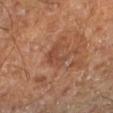{"biopsy_status": "not biopsied; imaged during a skin examination", "automated_metrics": {"area_mm2_approx": 6.0, "shape_asymmetry": 0.5, "border_irregularity_0_10": 6.0, "peripheral_color_asymmetry": 0.5}, "patient": {"age_approx": 65}, "image": {"source": "total-body photography crop", "field_of_view_mm": 15}, "site": "left lower leg", "lighting": "cross-polarized", "lesion_size": {"long_diameter_mm_approx": 3.5}}